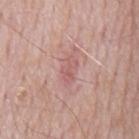The lesion was tiled from a total-body skin photograph and was not biopsied. The tile uses white-light illumination. Cropped from a whole-body photographic skin survey; the tile spans about 15 mm. The subject is a male aged 63–67. The lesion's longest dimension is about 3 mm. On the mid back.